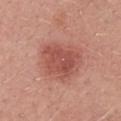The lesion was tiled from a total-body skin photograph and was not biopsied. The lesion is on the chest. The recorded lesion diameter is about 4.5 mm. A female patient, about 25 years old. A 15 mm crop from a total-body photograph taken for skin-cancer surveillance. The tile uses white-light illumination.